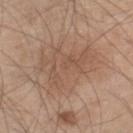Recorded during total-body skin imaging; not selected for excision or biopsy.
The recorded lesion diameter is about 8 mm.
A male patient about 45 years old.
The lesion is located on the right lower leg.
This is a white-light tile.
A 15 mm close-up tile from a total-body photography series done for melanoma screening.
The lesion-visualizer software estimated a lesion area of about 29 mm² and a symmetry-axis asymmetry near 0.4.